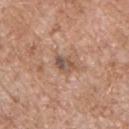biopsy status: catalogued during a skin exam; not biopsied
lighting: white-light illumination
automated lesion analysis: border irregularity of about 2.5 on a 0–10 scale and a within-lesion color-variation index near 9/10
anatomic site: the chest
patient: male, approximately 55 years of age
image source: 15 mm crop, total-body photography